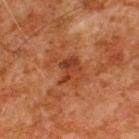biopsy_status: not biopsied; imaged during a skin examination
lesion_size:
  long_diameter_mm_approx: 4.0
site: back
automated_metrics:
  area_mm2_approx: 9.5
  eccentricity: 0.65
  shape_asymmetry: 0.45
  vs_skin_darker_L: 7.0
  vs_skin_contrast_norm: 6.5
  border_irregularity_0_10: 5.5
  color_variation_0_10: 4.0
  peripheral_color_asymmetry: 1.5
image:
  source: total-body photography crop
  field_of_view_mm: 15
patient:
  sex: male
  age_approx: 80
lighting: cross-polarized